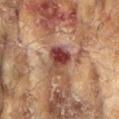A 15 mm crop from a total-body photograph taken for skin-cancer surveillance.
Automated tile analysis of the lesion measured an outline eccentricity of about 0.75 (0 = round, 1 = elongated) and two-axis asymmetry of about 0.4. And it measured border irregularity of about 4 on a 0–10 scale, internal color variation of about 9.5 on a 0–10 scale, and radial color variation of about 3.5.
A female subject, aged 78 to 82.
The tile uses cross-polarized illumination.
About 4 mm across.
From the arm.